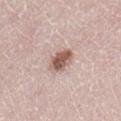Q: Was a biopsy performed?
A: total-body-photography surveillance lesion; no biopsy
Q: How large is the lesion?
A: ≈3 mm
Q: Who is the patient?
A: female, roughly 55 years of age
Q: What kind of image is this?
A: 15 mm crop, total-body photography
Q: How was the tile lit?
A: white-light
Q: Automated lesion metrics?
A: a lesion color around L≈57 a*≈18 b*≈25 in CIELAB and a lesion-to-skin contrast of about 9.5 (normalized; higher = more distinct); a border-irregularity index near 2/10
Q: Where on the body is the lesion?
A: the leg